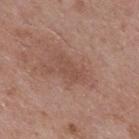– workup — total-body-photography surveillance lesion; no biopsy